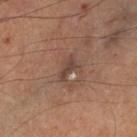automated metrics — a lesion area of about 3 mm² and an outline eccentricity of about 0.9 (0 = round, 1 = elongated); an automated nevus-likeness rating near 0 out of 100 and a detector confidence of about 75 out of 100 that the crop contains a lesion
image source — total-body-photography crop, ~15 mm field of view
subject — male, in their mid- to late 60s
location — the left lower leg
lesion diameter — ≈3 mm
tile lighting — cross-polarized illumination